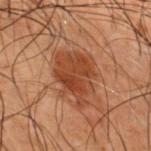Q: Was this lesion biopsied?
A: total-body-photography surveillance lesion; no biopsy
Q: Lesion location?
A: the upper back
Q: What kind of image is this?
A: total-body-photography crop, ~15 mm field of view
Q: Lesion size?
A: ~6 mm (longest diameter)
Q: How was the tile lit?
A: cross-polarized
Q: Patient demographics?
A: male, aged approximately 50
Q: Automated lesion metrics?
A: a footprint of about 17 mm², an eccentricity of roughly 0.65, and a shape-asymmetry score of about 0.3 (0 = symmetric); border irregularity of about 4 on a 0–10 scale and radial color variation of about 1